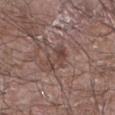Part of a total-body skin-imaging series; this lesion was reviewed on a skin check and was not flagged for biopsy. A lesion tile, about 15 mm wide, cut from a 3D total-body photograph. Captured under white-light illumination. A male patient aged approximately 80. Automated image analysis of the tile measured an area of roughly 5 mm², an eccentricity of roughly 0.9, and a shape-asymmetry score of about 0.55 (0 = symmetric). And it measured a detector confidence of about 95 out of 100 that the crop contains a lesion. The recorded lesion diameter is about 4 mm. On the right forearm.